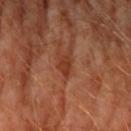This lesion was catalogued during total-body skin photography and was not selected for biopsy. Imaged with cross-polarized lighting. A 15 mm crop from a total-body photograph taken for skin-cancer surveillance. On the left upper arm. The lesion's longest dimension is about 2.5 mm. A male patient, aged approximately 60.